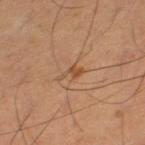Case summary:
• biopsy status — no biopsy performed (imaged during a skin exam)
• acquisition — total-body-photography crop, ~15 mm field of view
• size — ≈3 mm
• lighting — cross-polarized
• location — the leg
• subject — male, aged 58 to 62